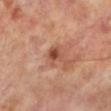Findings:
* workup: catalogued during a skin exam; not biopsied
* site: the left lower leg
* subject: male, aged around 70
* imaging modality: ~15 mm tile from a whole-body skin photo
* diameter: ~2.5 mm (longest diameter)
* illumination: cross-polarized
* automated lesion analysis: an average lesion color of about L≈49 a*≈23 b*≈31 (CIELAB), a lesion–skin lightness drop of about 10, and a normalized border contrast of about 7.5; peripheral color asymmetry of about 1.5; an automated nevus-likeness rating near 5 out of 100 and a lesion-detection confidence of about 100/100